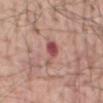Notes:
– notes · total-body-photography surveillance lesion; no biopsy
– size · about 3.5 mm
– acquisition · ~15 mm tile from a whole-body skin photo
– anatomic site · the abdomen
– illumination · white-light
– TBP lesion metrics · a nevus-likeness score of about 5/100 and lesion-presence confidence of about 100/100
– patient · male, in their 80s